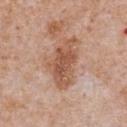No biopsy was performed on this lesion — it was imaged during a full skin examination and was not determined to be concerning. This is a white-light tile. Automated image analysis of the tile measured an average lesion color of about L≈55 a*≈22 b*≈31 (CIELAB) and a normalized lesion–skin contrast near 8. And it measured an automated nevus-likeness rating near 0 out of 100. A 15 mm crop from a total-body photograph taken for skin-cancer surveillance. Located on the chest. About 6.5 mm across. The subject is a male in their mid- to late 60s.